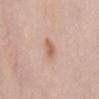notes: no biopsy performed (imaged during a skin exam)
subject: female, aged 48–52
imaging modality: ~15 mm tile from a whole-body skin photo
anatomic site: the mid back